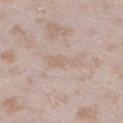Impression: No biopsy was performed on this lesion — it was imaged during a full skin examination and was not determined to be concerning. Context: A 15 mm close-up tile from a total-body photography series done for melanoma screening. The subject is a female in their mid-20s. Automated tile analysis of the lesion measured a footprint of about 4.5 mm², an outline eccentricity of about 0.95 (0 = round, 1 = elongated), and a symmetry-axis asymmetry near 0.45. The analysis additionally found a normalized border contrast of about 4.5. And it measured a border-irregularity index near 5.5/10. The recorded lesion diameter is about 4 mm. Located on the left lower leg. Imaged with white-light lighting.